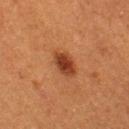| key | value |
|---|---|
| notes | imaged on a skin check; not biopsied |
| image | total-body-photography crop, ~15 mm field of view |
| patient | female, aged 38 to 42 |
| site | the left thigh |
| lesion size | ~3.5 mm (longest diameter) |
| tile lighting | cross-polarized |